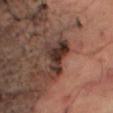Findings:
• notes: catalogued during a skin exam; not biopsied
• acquisition: total-body-photography crop, ~15 mm field of view
• body site: the right thigh
• tile lighting: cross-polarized
• patient: male, aged around 65
• automated metrics: a classifier nevus-likeness of about 0/100 and lesion-presence confidence of about 85/100
• diameter: ~5.5 mm (longest diameter)